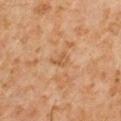Impression: The lesion was photographed on a routine skin check and not biopsied; there is no pathology result. Clinical summary: Located on the right upper arm. A 15 mm close-up extracted from a 3D total-body photography capture. A male subject aged 58–62. The total-body-photography lesion software estimated a footprint of about 3 mm² and an outline eccentricity of about 0.75 (0 = round, 1 = elongated). The software also gave a mean CIELAB color near L≈53 a*≈20 b*≈36, a lesion–skin lightness drop of about 7, and a normalized lesion–skin contrast near 5.5. The analysis additionally found a border-irregularity index near 5/10, internal color variation of about 2 on a 0–10 scale, and a peripheral color-asymmetry measure near 1. The analysis additionally found a classifier nevus-likeness of about 0/100 and lesion-presence confidence of about 100/100. The recorded lesion diameter is about 2.5 mm.